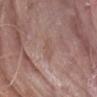Assessment: This lesion was catalogued during total-body skin photography and was not selected for biopsy. Acquisition and patient details: A male subject, aged 73 to 77. About 3 mm across. A 15 mm crop from a total-body photograph taken for skin-cancer surveillance. The lesion is located on the arm. An algorithmic analysis of the crop reported a lesion color around L≈53 a*≈18 b*≈25 in CIELAB and a normalized lesion–skin contrast near 4.5. Imaged with white-light lighting.